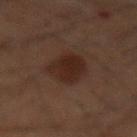Assessment: The lesion was tiled from a total-body skin photograph and was not biopsied. Clinical summary: The lesion is located on the mid back. A male patient, aged around 60. An algorithmic analysis of the crop reported an area of roughly 12 mm², an eccentricity of roughly 0.65, and a shape-asymmetry score of about 0.2 (0 = symmetric). It also reported a border-irregularity rating of about 2/10 and radial color variation of about 1. A 15 mm close-up extracted from a 3D total-body photography capture. Imaged with cross-polarized lighting.